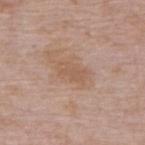This lesion was catalogued during total-body skin photography and was not selected for biopsy. A 15 mm crop from a total-body photograph taken for skin-cancer surveillance. The lesion is on the upper back. A male patient, in their mid-60s. About 3.5 mm across. Captured under white-light illumination.